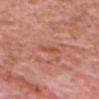<lesion>
  <biopsy_status>not biopsied; imaged during a skin examination</biopsy_status>
  <site>head or neck</site>
  <lesion_size>
    <long_diameter_mm_approx>2.5</long_diameter_mm_approx>
  </lesion_size>
  <patient>
    <sex>male</sex>
    <age_approx>80</age_approx>
  </patient>
  <image>
    <source>total-body photography crop</source>
    <field_of_view_mm>15</field_of_view_mm>
  </image>
</lesion>